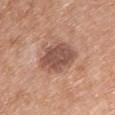This lesion was catalogued during total-body skin photography and was not selected for biopsy. A roughly 15 mm field-of-view crop from a total-body skin photograph. A female patient aged around 60. About 5.5 mm across. Captured under white-light illumination. On the chest.